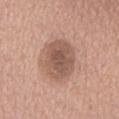biopsy status: no biopsy performed (imaged during a skin exam); size: ≈6 mm; anatomic site: the chest; tile lighting: white-light illumination; image source: total-body-photography crop, ~15 mm field of view; subject: male, in their mid- to late 60s.